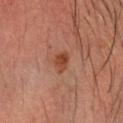<lesion>
<biopsy_status>not biopsied; imaged during a skin examination</biopsy_status>
<image>
  <source>total-body photography crop</source>
  <field_of_view_mm>15</field_of_view_mm>
</image>
<patient>
  <sex>male</sex>
  <age_approx>50</age_approx>
</patient>
<site>head or neck</site>
<lesion_size>
  <long_diameter_mm_approx>2.5</long_diameter_mm_approx>
</lesion_size>
<automated_metrics>
  <nevus_likeness_0_100>85</nevus_likeness_0_100>
</automated_metrics>
</lesion>